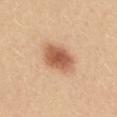Captured during whole-body skin photography for melanoma surveillance; the lesion was not biopsied.
This image is a 15 mm lesion crop taken from a total-body photograph.
The lesion is located on the mid back.
A female subject, aged around 25.
Longest diameter approximately 5 mm.
Automated tile analysis of the lesion measured a footprint of about 12 mm², an outline eccentricity of about 0.8 (0 = round, 1 = elongated), and two-axis asymmetry of about 0.2. The analysis additionally found a border-irregularity rating of about 2/10, a color-variation rating of about 4/10, and peripheral color asymmetry of about 1. It also reported a nevus-likeness score of about 100/100 and a detector confidence of about 100 out of 100 that the crop contains a lesion.
Imaged with white-light lighting.